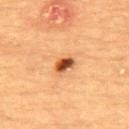| feature | finding |
|---|---|
| follow-up | catalogued during a skin exam; not biopsied |
| illumination | cross-polarized illumination |
| subject | female, aged 68 to 72 |
| location | the back |
| acquisition | total-body-photography crop, ~15 mm field of view |
| lesion size | about 2.5 mm |